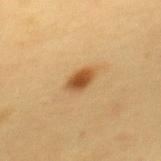Q: Was a biopsy performed?
A: no biopsy performed (imaged during a skin exam)
Q: Where on the body is the lesion?
A: the back
Q: How was the tile lit?
A: cross-polarized illumination
Q: What kind of image is this?
A: ~15 mm crop, total-body skin-cancer survey
Q: Who is the patient?
A: female, roughly 30 years of age
Q: Lesion size?
A: ~3 mm (longest diameter)